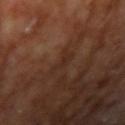No biopsy was performed on this lesion — it was imaged during a full skin examination and was not determined to be concerning. The tile uses cross-polarized illumination. The recorded lesion diameter is about 3 mm. Located on the right upper arm. A male patient, in their mid-50s. A 15 mm close-up extracted from a 3D total-body photography capture. The total-body-photography lesion software estimated a mean CIELAB color near L≈27 a*≈18 b*≈24 and roughly 4 lightness units darker than nearby skin. And it measured a lesion-detection confidence of about 85/100.